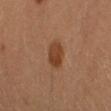Case summary:
* follow-up · total-body-photography surveillance lesion; no biopsy
* lighting · cross-polarized
* automated metrics · an outline eccentricity of about 0.55 (0 = round, 1 = elongated) and a shape-asymmetry score of about 0.15 (0 = symmetric); a border-irregularity index near 1.5/10, a color-variation rating of about 2.5/10, and a peripheral color-asymmetry measure near 1; a nevus-likeness score of about 95/100 and a lesion-detection confidence of about 100/100
* subject · female, aged approximately 30
* anatomic site · the left arm
* acquisition · ~15 mm tile from a whole-body skin photo